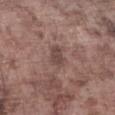biopsy status: catalogued during a skin exam; not biopsied | anatomic site: the leg | lesion diameter: ~4 mm (longest diameter) | lighting: white-light | image source: ~15 mm tile from a whole-body skin photo | subject: male, aged approximately 75.